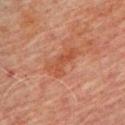Assessment: Captured during whole-body skin photography for melanoma surveillance; the lesion was not biopsied. Acquisition and patient details: The patient is a male about 70 years old. Longest diameter approximately 4.5 mm. A region of skin cropped from a whole-body photographic capture, roughly 15 mm wide. The lesion is on the upper back.